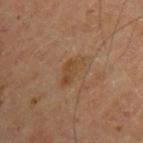Part of a total-body skin-imaging series; this lesion was reviewed on a skin check and was not flagged for biopsy.
The lesion is on the arm.
A roughly 15 mm field-of-view crop from a total-body skin photograph.
Captured under cross-polarized illumination.
A male patient in their mid- to late 60s.